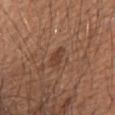No biopsy was performed on this lesion — it was imaged during a full skin examination and was not determined to be concerning. Located on the abdomen. About 2.5 mm across. A male patient, roughly 65 years of age. Captured under white-light illumination. This image is a 15 mm lesion crop taken from a total-body photograph.